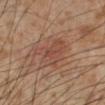The lesion was photographed on a routine skin check and not biopsied; there is no pathology result. The patient is a male aged approximately 55. A 15 mm crop from a total-body photograph taken for skin-cancer surveillance. The tile uses cross-polarized illumination. Automated tile analysis of the lesion measured a lesion area of about 11 mm² and a shape-asymmetry score of about 0.45 (0 = symmetric). The software also gave roughly 7 lightness units darker than nearby skin and a lesion-to-skin contrast of about 5.5 (normalized; higher = more distinct). And it measured border irregularity of about 5 on a 0–10 scale, internal color variation of about 3.5 on a 0–10 scale, and peripheral color asymmetry of about 1. It also reported an automated nevus-likeness rating near 30 out of 100. From the leg. About 5 mm across.